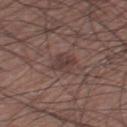Q: Is there a histopathology result?
A: imaged on a skin check; not biopsied
Q: What is the anatomic site?
A: the left thigh
Q: How was this image acquired?
A: 15 mm crop, total-body photography
Q: Who is the patient?
A: male, aged 58 to 62
Q: Illumination type?
A: white-light illumination
Q: What did automated image analysis measure?
A: a lesion area of about 5.5 mm²; an average lesion color of about L≈38 a*≈17 b*≈19 (CIELAB), a lesion–skin lightness drop of about 7, and a lesion-to-skin contrast of about 6.5 (normalized; higher = more distinct)